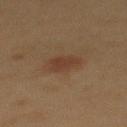workup: no biopsy performed (imaged during a skin exam); size: ≈3 mm; acquisition: total-body-photography crop, ~15 mm field of view; lighting: cross-polarized illumination; anatomic site: the back; subject: female, aged 48–52.